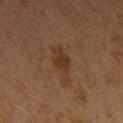Impression: Recorded during total-body skin imaging; not selected for excision or biopsy. Clinical summary: A region of skin cropped from a whole-body photographic capture, roughly 15 mm wide. Imaged with cross-polarized lighting. The lesion is on the left upper arm. A female patient aged 18 to 22. The recorded lesion diameter is about 4 mm.